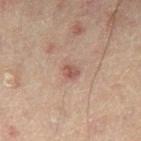Part of a total-body skin-imaging series; this lesion was reviewed on a skin check and was not flagged for biopsy. A male patient aged approximately 60. A 15 mm close-up extracted from a 3D total-body photography capture. On the left thigh. The lesion's longest dimension is about 2.5 mm.